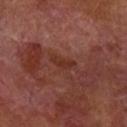Clinical impression: The lesion was photographed on a routine skin check and not biopsied; there is no pathology result. Acquisition and patient details: Approximately 3 mm at its widest. A male patient, roughly 70 years of age. A close-up tile cropped from a whole-body skin photograph, about 15 mm across. Located on the right forearm. This is a cross-polarized tile.